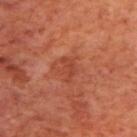Captured during whole-body skin photography for melanoma surveillance; the lesion was not biopsied. The recorded lesion diameter is about 3 mm. On the upper back. Automated tile analysis of the lesion measured a lesion area of about 3.5 mm² and an eccentricity of roughly 0.85. The analysis additionally found a mean CIELAB color near L≈43 a*≈31 b*≈33, about 6 CIELAB-L* units darker than the surrounding skin, and a normalized border contrast of about 5. The analysis additionally found border irregularity of about 5 on a 0–10 scale, a color-variation rating of about 1/10, and radial color variation of about 0.5. A male subject aged approximately 70. Imaged with cross-polarized lighting. A 15 mm crop from a total-body photograph taken for skin-cancer surveillance.